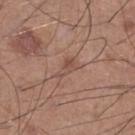notes: imaged on a skin check; not biopsied
illumination: white-light
subject: male, roughly 60 years of age
diameter: about 3 mm
imaging modality: ~15 mm crop, total-body skin-cancer survey
site: the leg